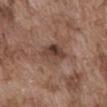No biopsy was performed on this lesion — it was imaged during a full skin examination and was not determined to be concerning. A lesion tile, about 15 mm wide, cut from a 3D total-body photograph. The recorded lesion diameter is about 3.5 mm. An algorithmic analysis of the crop reported a lesion–skin lightness drop of about 10. Located on the abdomen. The patient is a male aged around 75.